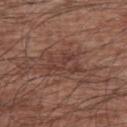Clinical impression:
No biopsy was performed on this lesion — it was imaged during a full skin examination and was not determined to be concerning.
Clinical summary:
A 15 mm close-up extracted from a 3D total-body photography capture. Captured under white-light illumination. Measured at roughly 4.5 mm in maximum diameter. A male patient aged 63 to 67. Automated image analysis of the tile measured an eccentricity of roughly 0.8 and a shape-asymmetry score of about 0.4 (0 = symmetric). The software also gave a border-irregularity rating of about 5/10, a within-lesion color-variation index near 4/10, and radial color variation of about 1.5. Located on the left upper arm.